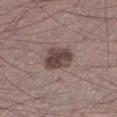The lesion was photographed on a routine skin check and not biopsied; there is no pathology result. The total-body-photography lesion software estimated a mean CIELAB color near L≈43 a*≈16 b*≈18, a lesion–skin lightness drop of about 13, and a normalized border contrast of about 10. It also reported a nevus-likeness score of about 50/100 and a detector confidence of about 100 out of 100 that the crop contains a lesion. Imaged with white-light lighting. Located on the left lower leg. A male subject aged 33 to 37. Measured at roughly 4 mm in maximum diameter. A region of skin cropped from a whole-body photographic capture, roughly 15 mm wide.